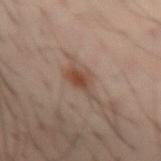Q: Was this lesion biopsied?
A: imaged on a skin check; not biopsied
Q: What is the imaging modality?
A: 15 mm crop, total-body photography
Q: Automated lesion metrics?
A: a peripheral color-asymmetry measure near 1.5
Q: Who is the patient?
A: male, aged around 40
Q: What is the anatomic site?
A: the arm